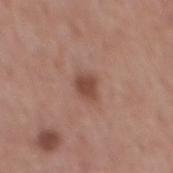Q: Was a biopsy performed?
A: catalogued during a skin exam; not biopsied
Q: Lesion location?
A: the mid back
Q: Who is the patient?
A: male, approximately 55 years of age
Q: What is the imaging modality?
A: ~15 mm tile from a whole-body skin photo